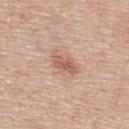The lesion was tiled from a total-body skin photograph and was not biopsied. On the upper back. This is a white-light tile. Measured at roughly 3.5 mm in maximum diameter. A male subject, aged around 55. A 15 mm close-up tile from a total-body photography series done for melanoma screening. Automated image analysis of the tile measured an eccentricity of roughly 0.8. It also reported a mean CIELAB color near L≈59 a*≈21 b*≈29, roughly 10 lightness units darker than nearby skin, and a normalized border contrast of about 7. It also reported a border-irregularity index near 3/10, a within-lesion color-variation index near 2.5/10, and radial color variation of about 1. It also reported an automated nevus-likeness rating near 65 out of 100 and a lesion-detection confidence of about 100/100.